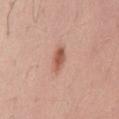Part of a total-body skin-imaging series; this lesion was reviewed on a skin check and was not flagged for biopsy.
The lesion's longest dimension is about 3 mm.
The tile uses white-light illumination.
The subject is a male aged approximately 30.
The lesion is located on the mid back.
Automated image analysis of the tile measured a footprint of about 4.5 mm² and an eccentricity of roughly 0.85. And it measured a mean CIELAB color near L≈56 a*≈25 b*≈30, a lesion–skin lightness drop of about 12, and a normalized border contrast of about 8.5. And it measured border irregularity of about 2 on a 0–10 scale and a color-variation rating of about 3/10.
A roughly 15 mm field-of-view crop from a total-body skin photograph.